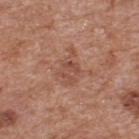workup=imaged on a skin check; not biopsied | acquisition=15 mm crop, total-body photography | anatomic site=the upper back | subject=male, roughly 65 years of age.